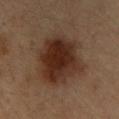Notes:
- biopsy status: total-body-photography surveillance lesion; no biopsy
- location: the upper back
- lighting: cross-polarized illumination
- image: ~15 mm tile from a whole-body skin photo
- lesion size: about 7.5 mm
- TBP lesion metrics: about 11 CIELAB-L* units darker than the surrounding skin and a normalized border contrast of about 11.5; a classifier nevus-likeness of about 95/100 and a lesion-detection confidence of about 100/100
- patient: male, in their mid-30s